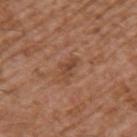Clinical impression:
Captured during whole-body skin photography for melanoma surveillance; the lesion was not biopsied.
Background:
Located on the left upper arm. A close-up tile cropped from a whole-body skin photograph, about 15 mm across. The total-body-photography lesion software estimated a lesion area of about 3 mm², an outline eccentricity of about 0.85 (0 = round, 1 = elongated), and a shape-asymmetry score of about 0.55 (0 = symmetric). It also reported border irregularity of about 6 on a 0–10 scale and peripheral color asymmetry of about 0. Measured at roughly 3 mm in maximum diameter. A male patient aged 73 to 77. Imaged with white-light lighting.